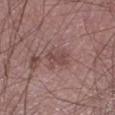Assessment:
The lesion was photographed on a routine skin check and not biopsied; there is no pathology result.
Image and clinical context:
Approximately 3.5 mm at its widest. A lesion tile, about 15 mm wide, cut from a 3D total-body photograph. The lesion is on the left lower leg. The subject is a male aged around 60. Captured under white-light illumination.